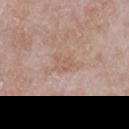– notes · catalogued during a skin exam; not biopsied
– tile lighting · white-light illumination
– subject · male, in their mid-60s
– lesion diameter · ≈2.5 mm
– imaging modality · 15 mm crop, total-body photography
– location · the right upper arm
– automated lesion analysis · a lesion color around L≈58 a*≈19 b*≈26 in CIELAB, about 6 CIELAB-L* units darker than the surrounding skin, and a normalized lesion–skin contrast near 4.5; border irregularity of about 4.5 on a 0–10 scale, a color-variation rating of about 1.5/10, and radial color variation of about 0.5